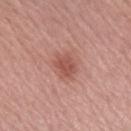Part of a total-body skin-imaging series; this lesion was reviewed on a skin check and was not flagged for biopsy.
The recorded lesion diameter is about 3 mm.
Located on the right forearm.
This is a white-light tile.
Cropped from a whole-body photographic skin survey; the tile spans about 15 mm.
The lesion-visualizer software estimated a border-irregularity rating of about 2/10, a color-variation rating of about 2/10, and radial color variation of about 1. The analysis additionally found a classifier nevus-likeness of about 65/100 and lesion-presence confidence of about 100/100.
The patient is a female aged approximately 65.